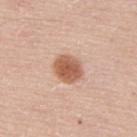<lesion>
  <biopsy_status>not biopsied; imaged during a skin examination</biopsy_status>
  <patient>
    <sex>male</sex>
    <age_approx>60</age_approx>
  </patient>
  <image>
    <source>total-body photography crop</source>
    <field_of_view_mm>15</field_of_view_mm>
  </image>
  <site>upper back</site>
  <automated_metrics>
    <area_mm2_approx>8.5</area_mm2_approx>
    <shape_asymmetry>0.15</shape_asymmetry>
    <cielab_L>58</cielab_L>
    <cielab_a>23</cielab_a>
    <cielab_b>32</cielab_b>
    <vs_skin_darker_L>14.0</vs_skin_darker_L>
    <vs_skin_contrast_norm>9.5</vs_skin_contrast_norm>
    <border_irregularity_0_10>1.5</border_irregularity_0_10>
    <color_variation_0_10>3.5</color_variation_0_10>
    <peripheral_color_asymmetry>1.0</peripheral_color_asymmetry>
    <nevus_likeness_0_100>100</nevus_likeness_0_100>
    <lesion_detection_confidence_0_100>100</lesion_detection_confidence_0_100>
  </automated_metrics>
  <lighting>white-light</lighting>
</lesion>